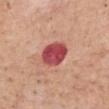follow-up: no biopsy performed (imaged during a skin exam) | body site: the mid back | lesion diameter: ≈3.5 mm | tile lighting: white-light illumination | patient: male, aged approximately 65 | imaging modality: ~15 mm tile from a whole-body skin photo.